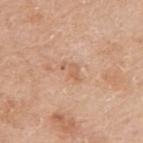The lesion was photographed on a routine skin check and not biopsied; there is no pathology result. On the right upper arm. The total-body-photography lesion software estimated an automated nevus-likeness rating near 0 out of 100 and lesion-presence confidence of about 100/100. A lesion tile, about 15 mm wide, cut from a 3D total-body photograph. The subject is a male about 60 years old. Captured under white-light illumination.